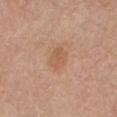biopsy_status: not biopsied; imaged during a skin examination
patient:
  sex: male
  age_approx: 80
automated_metrics:
  cielab_L: 57
  cielab_a: 21
  cielab_b: 32
  vs_skin_darker_L: 7.0
  vs_skin_contrast_norm: 5.0
  border_irregularity_0_10: 2.0
  color_variation_0_10: 1.5
  peripheral_color_asymmetry: 0.5
  lesion_detection_confidence_0_100: 100
image:
  source: total-body photography crop
  field_of_view_mm: 15
site: mid back